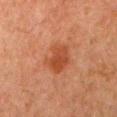biopsy_status: not biopsied; imaged during a skin examination
lesion_size:
  long_diameter_mm_approx: 4.0
patient:
  sex: male
  age_approx: 80
site: mid back
image:
  source: total-body photography crop
  field_of_view_mm: 15